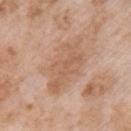The lesion was tiled from a total-body skin photograph and was not biopsied.
A 15 mm close-up extracted from a 3D total-body photography capture.
Imaged with white-light lighting.
Located on the left upper arm.
The lesion-visualizer software estimated an area of roughly 20 mm², a shape eccentricity near 0.85, and a symmetry-axis asymmetry near 0.25. It also reported a nevus-likeness score of about 0/100 and lesion-presence confidence of about 100/100.
Longest diameter approximately 7.5 mm.
A female subject aged 68–72.